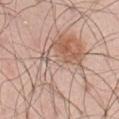Recorded during total-body skin imaging; not selected for excision or biopsy.
Approximately 9.5 mm at its widest.
This image is a 15 mm lesion crop taken from a total-body photograph.
The subject is a male aged approximately 55.
The lesion is on the abdomen.